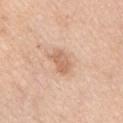Recorded during total-body skin imaging; not selected for excision or biopsy.
This is a white-light tile.
The patient is a female approximately 55 years of age.
The lesion's longest dimension is about 3 mm.
This image is a 15 mm lesion crop taken from a total-body photograph.
From the left upper arm.
An algorithmic analysis of the crop reported a footprint of about 5 mm² and a shape-asymmetry score of about 0.25 (0 = symmetric). The analysis additionally found a mean CIELAB color near L≈64 a*≈21 b*≈32, about 10 CIELAB-L* units darker than the surrounding skin, and a normalized lesion–skin contrast near 6.5. It also reported border irregularity of about 2.5 on a 0–10 scale and radial color variation of about 1. The analysis additionally found a detector confidence of about 100 out of 100 that the crop contains a lesion.